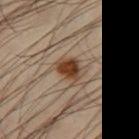Clinical impression:
This lesion was catalogued during total-body skin photography and was not selected for biopsy.
Clinical summary:
A roughly 15 mm field-of-view crop from a total-body skin photograph. The lesion is located on the right thigh. A male subject, roughly 50 years of age.